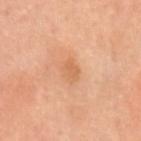Q: Was a biopsy performed?
A: imaged on a skin check; not biopsied
Q: Lesion size?
A: about 2.5 mm
Q: What lighting was used for the tile?
A: cross-polarized illumination
Q: Automated lesion metrics?
A: a footprint of about 4 mm² and two-axis asymmetry of about 0.2; an average lesion color of about L≈64 a*≈24 b*≈39 (CIELAB) and roughly 7 lightness units darker than nearby skin; a border-irregularity rating of about 2/10, a color-variation rating of about 2.5/10, and radial color variation of about 1
Q: How was this image acquired?
A: total-body-photography crop, ~15 mm field of view
Q: What are the patient's age and sex?
A: male, aged 38 to 42
Q: Lesion location?
A: the head or neck